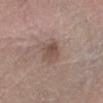This lesion was catalogued during total-body skin photography and was not selected for biopsy. The subject is a male about 75 years old. Automated image analysis of the tile measured a lesion area of about 5 mm² and a symmetry-axis asymmetry near 0.25. It also reported an average lesion color of about L≈49 a*≈17 b*≈23 (CIELAB), roughly 9 lightness units darker than nearby skin, and a lesion-to-skin contrast of about 7 (normalized; higher = more distinct). The software also gave a border-irregularity index near 2.5/10, internal color variation of about 3.5 on a 0–10 scale, and radial color variation of about 1. The analysis additionally found a classifier nevus-likeness of about 15/100 and a lesion-detection confidence of about 100/100. The lesion is located on the right forearm. Longest diameter approximately 2.5 mm. Captured under white-light illumination. This image is a 15 mm lesion crop taken from a total-body photograph.